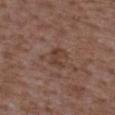The lesion was photographed on a routine skin check and not biopsied; there is no pathology result.
The total-body-photography lesion software estimated a lesion area of about 5 mm², an eccentricity of roughly 0.6, and two-axis asymmetry of about 0.35. And it measured a classifier nevus-likeness of about 0/100 and a detector confidence of about 100 out of 100 that the crop contains a lesion.
From the upper back.
A 15 mm close-up extracted from a 3D total-body photography capture.
The lesion's longest dimension is about 2.5 mm.
The patient is a male aged 48–52.